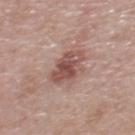notes: no biopsy performed (imaged during a skin exam)
lesion diameter: ~3.5 mm (longest diameter)
image-analysis metrics: a shape-asymmetry score of about 0.2 (0 = symmetric); a lesion color around L≈50 a*≈22 b*≈23 in CIELAB, roughly 12 lightness units darker than nearby skin, and a lesion-to-skin contrast of about 8 (normalized; higher = more distinct)
subject: male, aged 63 to 67
body site: the upper back
imaging modality: total-body-photography crop, ~15 mm field of view
tile lighting: white-light illumination